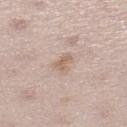lighting = white-light
body site = the left lower leg
image source = ~15 mm crop, total-body skin-cancer survey
patient = female, aged 48–52
image-analysis metrics = a border-irregularity index near 4.5/10 and radial color variation of about 0.5; a detector confidence of about 100 out of 100 that the crop contains a lesion
diameter = about 3 mm